The total-body-photography lesion software estimated an area of roughly 21 mm², a shape eccentricity near 0.75, and a shape-asymmetry score of about 0.3 (0 = symmetric). The software also gave an average lesion color of about L≈55 a*≈28 b*≈29 (CIELAB), a lesion–skin lightness drop of about 8, and a normalized lesion–skin contrast near 6. The analysis additionally found lesion-presence confidence of about 100/100; a male patient about 45 years old; a region of skin cropped from a whole-body photographic capture, roughly 15 mm wide; located on the right lower leg:
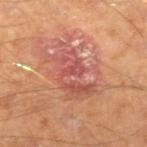Diagnosis:
On biopsy, histopathology showed a squamous cell carcinoma in situ, classified as a skin cancer.